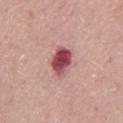Recorded during total-body skin imaging; not selected for excision or biopsy. A male subject, approximately 70 years of age. The lesion is located on the abdomen. A 15 mm close-up extracted from a 3D total-body photography capture.